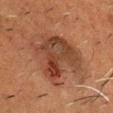| field | value |
|---|---|
| biopsy status | no biopsy performed (imaged during a skin exam) |
| lighting | cross-polarized |
| subject | male, about 75 years old |
| lesion size | ≈7 mm |
| site | the head or neck |
| acquisition | ~15 mm tile from a whole-body skin photo |